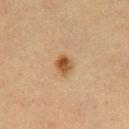Assessment:
The lesion was tiled from a total-body skin photograph and was not biopsied.
Clinical summary:
A male subject aged around 50. Located on the chest. A roughly 15 mm field-of-view crop from a total-body skin photograph.